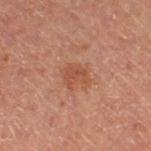biopsy_status: not biopsied; imaged during a skin examination
site: right thigh
patient:
  sex: female
lighting: cross-polarized
image:
  source: total-body photography crop
  field_of_view_mm: 15